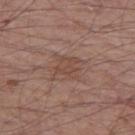Recorded during total-body skin imaging; not selected for excision or biopsy. Located on the left thigh. A close-up tile cropped from a whole-body skin photograph, about 15 mm across. The lesion's longest dimension is about 3 mm. A male patient, in their mid-50s.